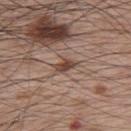  biopsy_status: not biopsied; imaged during a skin examination
  patient:
    sex: male
    age_approx: 65
  site: upper back
  image:
    source: total-body photography crop
    field_of_view_mm: 15